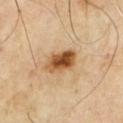Q: Was this lesion biopsied?
A: imaged on a skin check; not biopsied
Q: Patient demographics?
A: male, in their mid-60s
Q: What is the imaging modality?
A: total-body-photography crop, ~15 mm field of view
Q: Where on the body is the lesion?
A: the chest